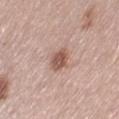Assessment: The lesion was photographed on a routine skin check and not biopsied; there is no pathology result. Image and clinical context: A 15 mm crop from a total-body photograph taken for skin-cancer surveillance. The tile uses white-light illumination. Located on the leg. A female subject, roughly 65 years of age.